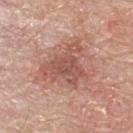| feature | finding |
|---|---|
| notes | total-body-photography surveillance lesion; no biopsy |
| tile lighting | white-light |
| anatomic site | the upper back |
| image source | ~15 mm crop, total-body skin-cancer survey |
| lesion size | about 6 mm |
| TBP lesion metrics | a footprint of about 17 mm² and a symmetry-axis asymmetry near 0.5; a within-lesion color-variation index near 3.5/10 and peripheral color asymmetry of about 1 |
| patient | male, aged approximately 70 |